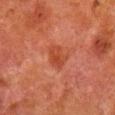Q: Was this lesion biopsied?
A: imaged on a skin check; not biopsied
Q: What is the lesion's diameter?
A: ≈3 mm
Q: What kind of image is this?
A: total-body-photography crop, ~15 mm field of view
Q: Automated lesion metrics?
A: a lesion area of about 5.5 mm², an outline eccentricity of about 0.65 (0 = round, 1 = elongated), and two-axis asymmetry of about 0.25
Q: Where on the body is the lesion?
A: the right lower leg
Q: Patient demographics?
A: male, about 80 years old